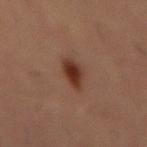follow-up = imaged on a skin check; not biopsied | patient = male, aged approximately 55 | lesion size = ≈3.5 mm | acquisition = ~15 mm tile from a whole-body skin photo | site = the abdomen | illumination = cross-polarized.